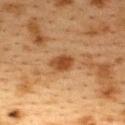Notes:
• follow-up: catalogued during a skin exam; not biopsied
• illumination: cross-polarized
• patient: female, aged around 40
• diameter: ~3 mm (longest diameter)
• location: the upper back
• automated metrics: an eccentricity of roughly 0.7 and a symmetry-axis asymmetry near 0.25; about 11 CIELAB-L* units darker than the surrounding skin and a normalized lesion–skin contrast near 9.5; an automated nevus-likeness rating near 75 out of 100 and a lesion-detection confidence of about 100/100
• image: 15 mm crop, total-body photography